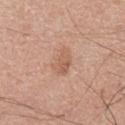Notes:
- follow-up: total-body-photography surveillance lesion; no biopsy
- TBP lesion metrics: an average lesion color of about L≈59 a*≈21 b*≈30 (CIELAB), roughly 8 lightness units darker than nearby skin, and a normalized lesion–skin contrast near 5.5
- lesion size: ≈3.5 mm
- imaging modality: ~15 mm crop, total-body skin-cancer survey
- site: the abdomen
- tile lighting: white-light
- subject: male, aged 73 to 77